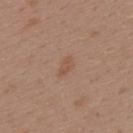lighting — white-light illumination
body site — the upper back
imaging modality — ~15 mm crop, total-body skin-cancer survey
image-analysis metrics — a lesion area of about 2.5 mm² and a symmetry-axis asymmetry near 0.3; an average lesion color of about L≈52 a*≈19 b*≈29 (CIELAB) and about 6 CIELAB-L* units darker than the surrounding skin; a border-irregularity rating of about 2.5/10, a color-variation rating of about 0.5/10, and peripheral color asymmetry of about 0; an automated nevus-likeness rating near 10 out of 100 and a lesion-detection confidence of about 100/100
lesion diameter — ≈2.5 mm
patient — female, aged approximately 40